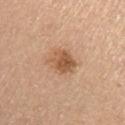This lesion was catalogued during total-body skin photography and was not selected for biopsy. The lesion is located on the right upper arm. The patient is a female in their mid-60s. Imaged with white-light lighting. A 15 mm close-up tile from a total-body photography series done for melanoma screening.